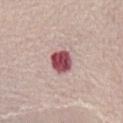Findings:
- illumination: white-light illumination
- TBP lesion metrics: a footprint of about 6.5 mm², an eccentricity of roughly 0.45, and a symmetry-axis asymmetry near 0.15; a lesion–skin lightness drop of about 21 and a lesion-to-skin contrast of about 13.5 (normalized; higher = more distinct); a nevus-likeness score of about 0/100 and lesion-presence confidence of about 100/100
- patient: female, in their mid-60s
- image: total-body-photography crop, ~15 mm field of view
- lesion size: ~3 mm (longest diameter)
- body site: the abdomen